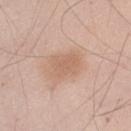Background: A male subject roughly 45 years of age. Cropped from a total-body skin-imaging series; the visible field is about 15 mm. The lesion is located on the left upper arm.